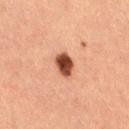{
  "biopsy_status": "not biopsied; imaged during a skin examination",
  "patient": {
    "sex": "female",
    "age_approx": 40
  },
  "lighting": "cross-polarized",
  "image": {
    "source": "total-body photography crop",
    "field_of_view_mm": 15
  },
  "lesion_size": {
    "long_diameter_mm_approx": 3.0
  },
  "site": "right thigh"
}